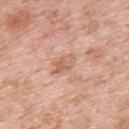{
  "biopsy_status": "not biopsied; imaged during a skin examination",
  "image": {
    "source": "total-body photography crop",
    "field_of_view_mm": 15
  },
  "patient": {
    "sex": "female",
    "age_approx": 50
  },
  "site": "back",
  "lesion_size": {
    "long_diameter_mm_approx": 3.5
  }
}